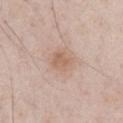<case>
<biopsy_status>not biopsied; imaged during a skin examination</biopsy_status>
<automated_metrics>
  <area_mm2_approx>5.0</area_mm2_approx>
  <eccentricity>0.55</eccentricity>
  <shape_asymmetry>0.25</shape_asymmetry>
  <border_irregularity_0_10>2.5</border_irregularity_0_10>
  <color_variation_0_10>2.0</color_variation_0_10>
  <peripheral_color_asymmetry>0.5</peripheral_color_asymmetry>
  <nevus_likeness_0_100>15</nevus_likeness_0_100>
</automated_metrics>
<lesion_size>
  <long_diameter_mm_approx>2.5</long_diameter_mm_approx>
</lesion_size>
<site>abdomen</site>
<patient>
  <sex>male</sex>
  <age_approx>60</age_approx>
</patient>
<lighting>white-light</lighting>
<image>
  <source>total-body photography crop</source>
  <field_of_view_mm>15</field_of_view_mm>
</image>
</case>